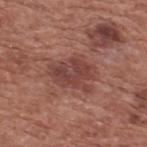Q: Was a biopsy performed?
A: catalogued during a skin exam; not biopsied
Q: What did automated image analysis measure?
A: an automated nevus-likeness rating near 0 out of 100
Q: What kind of image is this?
A: ~15 mm crop, total-body skin-cancer survey
Q: What is the anatomic site?
A: the upper back
Q: Who is the patient?
A: male, in their mid- to late 70s
Q: What is the lesion's diameter?
A: about 5.5 mm